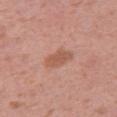The lesion was photographed on a routine skin check and not biopsied; there is no pathology result. Located on the right thigh. A female subject, aged approximately 40. A close-up tile cropped from a whole-body skin photograph, about 15 mm across. Longest diameter approximately 3.5 mm.